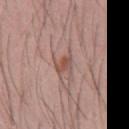The lesion was tiled from a total-body skin photograph and was not biopsied.